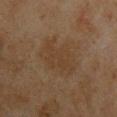Q: Is there a histopathology result?
A: imaged on a skin check; not biopsied
Q: What did automated image analysis measure?
A: a mean CIELAB color near L≈31 a*≈12 b*≈25; internal color variation of about 2 on a 0–10 scale and a peripheral color-asymmetry measure near 1
Q: How was this image acquired?
A: total-body-photography crop, ~15 mm field of view
Q: What is the lesion's diameter?
A: ≈5 mm
Q: Where on the body is the lesion?
A: the chest
Q: How was the tile lit?
A: cross-polarized illumination
Q: Who is the patient?
A: male, in their mid-40s